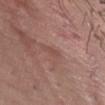follow-up — no biopsy performed (imaged during a skin exam); imaging modality — ~15 mm crop, total-body skin-cancer survey; lesion size — ~2.5 mm (longest diameter); tile lighting — white-light; body site — the front of the torso; patient — female, approximately 40 years of age; image-analysis metrics — a lesion area of about 2.5 mm², a shape eccentricity near 0.9, and a shape-asymmetry score of about 0.35 (0 = symmetric).